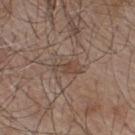Clinical impression: The lesion was photographed on a routine skin check and not biopsied; there is no pathology result. Acquisition and patient details: On the upper back. About 3.5 mm across. Cropped from a total-body skin-imaging series; the visible field is about 15 mm. A male patient, about 55 years old.